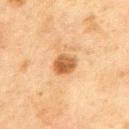From the back.
The subject is a male aged around 45.
The tile uses cross-polarized illumination.
Automated tile analysis of the lesion measured an area of roughly 5.5 mm². The software also gave a normalized lesion–skin contrast near 9.5. It also reported a border-irregularity index near 1.5/10, internal color variation of about 4.5 on a 0–10 scale, and radial color variation of about 1.5.
A lesion tile, about 15 mm wide, cut from a 3D total-body photograph.
Measured at roughly 3 mm in maximum diameter.